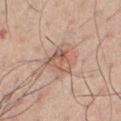This lesion was catalogued during total-body skin photography and was not selected for biopsy.
A 15 mm close-up tile from a total-body photography series done for melanoma screening.
On the chest.
Captured under white-light illumination.
A male subject aged around 60.
The lesion's longest dimension is about 3.5 mm.